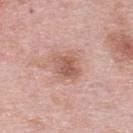Impression: No biopsy was performed on this lesion — it was imaged during a full skin examination and was not determined to be concerning. Clinical summary: Automated image analysis of the tile measured an area of roughly 7.5 mm². The software also gave a lesion color around L≈57 a*≈23 b*≈27 in CIELAB, roughly 10 lightness units darker than nearby skin, and a normalized lesion–skin contrast near 7. The software also gave a border-irregularity rating of about 2.5/10, internal color variation of about 4 on a 0–10 scale, and peripheral color asymmetry of about 1.5. The analysis additionally found a detector confidence of about 100 out of 100 that the crop contains a lesion. A female patient aged 63 to 67. A 15 mm crop from a total-body photograph taken for skin-cancer surveillance. Longest diameter approximately 3 mm. The lesion is on the back.